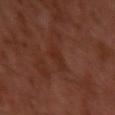biopsy status: total-body-photography surveillance lesion; no biopsy
image: ~15 mm tile from a whole-body skin photo
location: the arm
patient: male, aged around 30